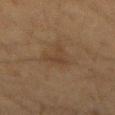notes = catalogued during a skin exam; not biopsied
illumination = cross-polarized
subject = male, aged around 60
acquisition = ~15 mm tile from a whole-body skin photo
site = the mid back